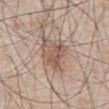Q: Was a biopsy performed?
A: no biopsy performed (imaged during a skin exam)
Q: Where on the body is the lesion?
A: the abdomen
Q: Patient demographics?
A: male, in their mid-60s
Q: How was this image acquired?
A: total-body-photography crop, ~15 mm field of view